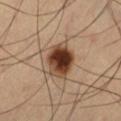Captured during whole-body skin photography for melanoma surveillance; the lesion was not biopsied.
The subject is a male in their mid- to late 60s.
The recorded lesion diameter is about 4.5 mm.
The lesion is located on the left thigh.
Cropped from a total-body skin-imaging series; the visible field is about 15 mm.
Imaged with cross-polarized lighting.